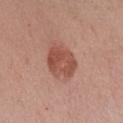| feature | finding |
|---|---|
| automated lesion analysis | a lesion color around L≈51 a*≈25 b*≈28 in CIELAB, about 11 CIELAB-L* units darker than the surrounding skin, and a normalized lesion–skin contrast near 7.5; border irregularity of about 1.5 on a 0–10 scale and a color-variation rating of about 4/10 |
| location | the left forearm |
| subject | female, aged around 40 |
| tile lighting | white-light |
| acquisition | total-body-photography crop, ~15 mm field of view |
| lesion diameter | about 4.5 mm |